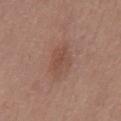Q: Was this lesion biopsied?
A: catalogued during a skin exam; not biopsied
Q: What are the patient's age and sex?
A: male, about 75 years old
Q: What is the imaging modality?
A: 15 mm crop, total-body photography
Q: What is the lesion's diameter?
A: about 4 mm
Q: What is the anatomic site?
A: the front of the torso
Q: What lighting was used for the tile?
A: white-light illumination